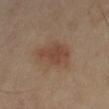Imaged with cross-polarized lighting.
About 4.5 mm across.
On the leg.
Cropped from a whole-body photographic skin survey; the tile spans about 15 mm.
The patient is a female aged around 70.